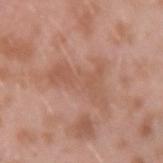  site: left forearm
  lighting: white-light
  image:
    source: total-body photography crop
    field_of_view_mm: 15
  patient:
    sex: male
    age_approx: 40
  lesion_size:
    long_diameter_mm_approx: 6.5